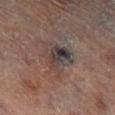<tbp_lesion>
<biopsy_status>not biopsied; imaged during a skin examination</biopsy_status>
<patient>
  <sex>female</sex>
  <age_approx>80</age_approx>
</patient>
<image>
  <source>total-body photography crop</source>
  <field_of_view_mm>15</field_of_view_mm>
</image>
<lesion_size>
  <long_diameter_mm_approx>5.0</long_diameter_mm_approx>
</lesion_size>
<site>left leg</site>
</tbp_lesion>